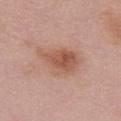The lesion was tiled from a total-body skin photograph and was not biopsied. Longest diameter approximately 6 mm. The subject is a female approximately 65 years of age. The lesion is on the chest. Imaged with white-light lighting. This image is a 15 mm lesion crop taken from a total-body photograph.